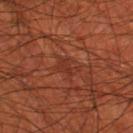Assessment: The lesion was photographed on a routine skin check and not biopsied; there is no pathology result. Background: A 15 mm close-up extracted from a 3D total-body photography capture. The subject is a male aged around 70. From the left thigh. Approximately 2.5 mm at its widest.